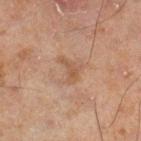workup=no biopsy performed (imaged during a skin exam) | patient=male, in their mid-40s | size=~3 mm (longest diameter) | image=15 mm crop, total-body photography | location=the right lower leg | lighting=cross-polarized.